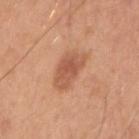patient = male, in their 30s; diameter = about 4.5 mm; tile lighting = white-light; image source = total-body-photography crop, ~15 mm field of view; body site = the right upper arm.